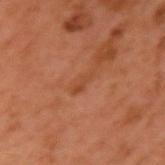Case summary:
* notes: no biopsy performed (imaged during a skin exam)
* diameter: ~3 mm (longest diameter)
* patient: male, in their 60s
* image: ~15 mm tile from a whole-body skin photo
* illumination: cross-polarized illumination
* body site: the left upper arm